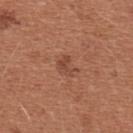workup: imaged on a skin check; not biopsied
automated metrics: a shape eccentricity near 0.65 and a symmetry-axis asymmetry near 0.4; an average lesion color of about L≈46 a*≈24 b*≈30 (CIELAB), roughly 7 lightness units darker than nearby skin, and a normalized border contrast of about 5.5; a border-irregularity rating of about 4/10, a color-variation rating of about 2.5/10, and peripheral color asymmetry of about 1; a lesion-detection confidence of about 100/100
body site: the front of the torso
lesion diameter: ~2.5 mm (longest diameter)
patient: female, aged around 35
image source: ~15 mm tile from a whole-body skin photo